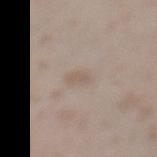{
  "biopsy_status": "not biopsied; imaged during a skin examination",
  "site": "leg",
  "image": {
    "source": "total-body photography crop",
    "field_of_view_mm": 15
  },
  "automated_metrics": {
    "area_mm2_approx": 3.0,
    "eccentricity": 0.9,
    "shape_asymmetry": 0.3,
    "cielab_L": 57,
    "cielab_a": 12,
    "cielab_b": 25,
    "vs_skin_darker_L": 6.0,
    "vs_skin_contrast_norm": 5.0,
    "nevus_likeness_0_100": 0,
    "lesion_detection_confidence_0_100": 95
  },
  "patient": {
    "sex": "female",
    "age_approx": 25
  },
  "lighting": "white-light"
}